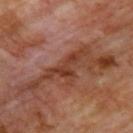No biopsy was performed on this lesion — it was imaged during a full skin examination and was not determined to be concerning. A 15 mm close-up tile from a total-body photography series done for melanoma screening. A male patient roughly 60 years of age. Measured at roughly 6 mm in maximum diameter. Imaged with cross-polarized lighting. From the upper back. Automated image analysis of the tile measured a shape eccentricity near 0.95 and a symmetry-axis asymmetry near 0.7. It also reported border irregularity of about 10 on a 0–10 scale, internal color variation of about 2 on a 0–10 scale, and radial color variation of about 0.5.